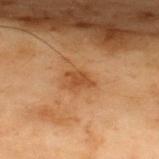Part of a total-body skin-imaging series; this lesion was reviewed on a skin check and was not flagged for biopsy. A male patient, approximately 55 years of age. A lesion tile, about 15 mm wide, cut from a 3D total-body photograph. On the upper back. The recorded lesion diameter is about 2.5 mm. The tile uses cross-polarized illumination. Automated image analysis of the tile measured a lesion color around L≈40 a*≈21 b*≈34 in CIELAB, a lesion–skin lightness drop of about 8, and a normalized border contrast of about 7. The software also gave an automated nevus-likeness rating near 35 out of 100 and a detector confidence of about 100 out of 100 that the crop contains a lesion.